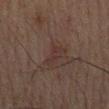The lesion was tiled from a total-body skin photograph and was not biopsied. A male subject, aged around 70. On the chest. A 15 mm crop from a total-body photograph taken for skin-cancer surveillance.